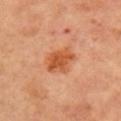Recorded during total-body skin imaging; not selected for excision or biopsy.
A female patient roughly 65 years of age.
About 4 mm across.
Automated tile analysis of the lesion measured an area of roughly 9.5 mm² and a symmetry-axis asymmetry near 0.25. The software also gave a color-variation rating of about 4/10 and peripheral color asymmetry of about 1.5.
From the arm.
Imaged with cross-polarized lighting.
A region of skin cropped from a whole-body photographic capture, roughly 15 mm wide.